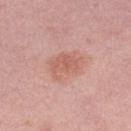biopsy status: imaged on a skin check; not biopsied
imaging modality: ~15 mm crop, total-body skin-cancer survey
diameter: ≈4 mm
location: the left lower leg
image-analysis metrics: a mean CIELAB color near L≈61 a*≈24 b*≈28, roughly 8 lightness units darker than nearby skin, and a normalized border contrast of about 5.5; border irregularity of about 2 on a 0–10 scale, internal color variation of about 2.5 on a 0–10 scale, and peripheral color asymmetry of about 1
patient: female, roughly 50 years of age
lighting: white-light illumination